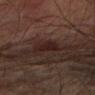Assessment: Captured during whole-body skin photography for melanoma surveillance; the lesion was not biopsied. Acquisition and patient details: A male subject, aged around 75. The lesion is located on the arm. Automated image analysis of the tile measured an average lesion color of about L≈16 a*≈15 b*≈16 (CIELAB), roughly 6 lightness units darker than nearby skin, and a normalized border contrast of about 8. And it measured a classifier nevus-likeness of about 5/100 and lesion-presence confidence of about 100/100. The tile uses cross-polarized illumination. This image is a 15 mm lesion crop taken from a total-body photograph. Longest diameter approximately 3 mm.